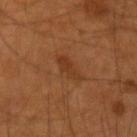Assessment: Recorded during total-body skin imaging; not selected for excision or biopsy. Image and clinical context: Imaged with cross-polarized lighting. On the right forearm. A roughly 15 mm field-of-view crop from a total-body skin photograph. The patient is a male in their mid- to late 50s. The recorded lesion diameter is about 3 mm.